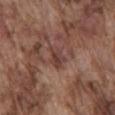Notes:
- biopsy status · total-body-photography surveillance lesion; no biopsy
- subject · male, roughly 75 years of age
- diameter · about 2.5 mm
- illumination · white-light illumination
- location · the mid back
- acquisition · 15 mm crop, total-body photography
- automated lesion analysis · a lesion area of about 3.5 mm² and a symmetry-axis asymmetry near 0.4